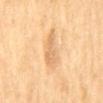{
  "biopsy_status": "not biopsied; imaged during a skin examination",
  "patient": {
    "sex": "male",
    "age_approx": 70
  },
  "site": "mid back",
  "lesion_size": {
    "long_diameter_mm_approx": 4.5
  },
  "automated_metrics": {
    "eccentricity": 0.9,
    "border_irregularity_0_10": 6.0,
    "peripheral_color_asymmetry": 0.5
  },
  "image": {
    "source": "total-body photography crop",
    "field_of_view_mm": 15
  },
  "lighting": "cross-polarized"
}